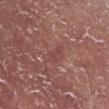This lesion was catalogued during total-body skin photography and was not selected for biopsy. From the right lower leg. Captured under white-light illumination. Longest diameter approximately 2.5 mm. The patient is a male aged around 55. Cropped from a total-body skin-imaging series; the visible field is about 15 mm.